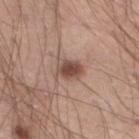  biopsy_status: not biopsied; imaged during a skin examination
  image:
    source: total-body photography crop
    field_of_view_mm: 15
  lighting: white-light
  lesion_size:
    long_diameter_mm_approx: 3.5
  patient:
    sex: male
    age_approx: 40
  site: leg